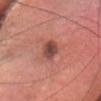Part of a total-body skin-imaging series; this lesion was reviewed on a skin check and was not flagged for biopsy.
This image is a 15 mm lesion crop taken from a total-body photograph.
From the head or neck.
Captured under white-light illumination.
About 3.5 mm across.
The patient is a female in their mid-50s.
An algorithmic analysis of the crop reported border irregularity of about 1.5 on a 0–10 scale, a within-lesion color-variation index near 3.5/10, and peripheral color asymmetry of about 1. It also reported an automated nevus-likeness rating near 10 out of 100 and a lesion-detection confidence of about 100/100.